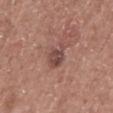Part of a total-body skin-imaging series; this lesion was reviewed on a skin check and was not flagged for biopsy. A 15 mm close-up tile from a total-body photography series done for melanoma screening. Imaged with white-light lighting. Located on the mid back. Automated tile analysis of the lesion measured a lesion area of about 5 mm² and a shape-asymmetry score of about 0.35 (0 = symmetric). It also reported a lesion color around L≈46 a*≈20 b*≈23 in CIELAB, about 10 CIELAB-L* units darker than the surrounding skin, and a normalized lesion–skin contrast near 7.5. And it measured a classifier nevus-likeness of about 5/100. A male patient about 60 years old.